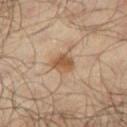Case summary:
- patient — male, in their mid-60s
- lesion diameter — ~3 mm (longest diameter)
- image source — 15 mm crop, total-body photography
- image-analysis metrics — an area of roughly 5.5 mm² and two-axis asymmetry of about 0.2; an average lesion color of about L≈50 a*≈17 b*≈32 (CIELAB) and a normalized border contrast of about 8; border irregularity of about 2 on a 0–10 scale and internal color variation of about 3.5 on a 0–10 scale; an automated nevus-likeness rating near 90 out of 100 and lesion-presence confidence of about 100/100
- illumination — cross-polarized illumination
- location — the right thigh